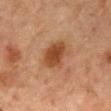acquisition: ~15 mm crop, total-body skin-cancer survey | anatomic site: the front of the torso | tile lighting: cross-polarized | subject: male, approximately 65 years of age | automated metrics: a border-irregularity index near 2.5/10, internal color variation of about 2.5 on a 0–10 scale, and radial color variation of about 0.5; an automated nevus-likeness rating near 95 out of 100 and lesion-presence confidence of about 100/100.